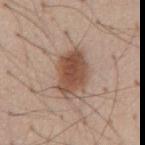follow-up = total-body-photography surveillance lesion; no biopsy | subject = male, in their mid-50s | acquisition = 15 mm crop, total-body photography | body site = the abdomen | lighting = white-light illumination.